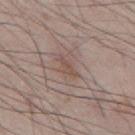Impression: Recorded during total-body skin imaging; not selected for excision or biopsy. Context: On the back. This is a white-light tile. A male subject aged around 35. The total-body-photography lesion software estimated a footprint of about 3 mm², a shape eccentricity near 0.9, and a symmetry-axis asymmetry near 0.45. The software also gave an average lesion color of about L≈50 a*≈16 b*≈23 (CIELAB). It also reported border irregularity of about 4.5 on a 0–10 scale and radial color variation of about 0. Approximately 3 mm at its widest. A 15 mm close-up tile from a total-body photography series done for melanoma screening.